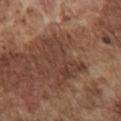Q: Was this lesion biopsied?
A: total-body-photography surveillance lesion; no biopsy
Q: What is the lesion's diameter?
A: about 6.5 mm
Q: What kind of image is this?
A: ~15 mm tile from a whole-body skin photo
Q: Automated lesion metrics?
A: a lesion–skin lightness drop of about 7; border irregularity of about 5.5 on a 0–10 scale and radial color variation of about 1; a nevus-likeness score of about 0/100 and a lesion-detection confidence of about 90/100
Q: Where on the body is the lesion?
A: the chest
Q: How was the tile lit?
A: white-light illumination
Q: What are the patient's age and sex?
A: male, about 75 years old